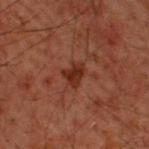Part of a total-body skin-imaging series; this lesion was reviewed on a skin check and was not flagged for biopsy. An algorithmic analysis of the crop reported an eccentricity of roughly 0.65 and a shape-asymmetry score of about 0.4 (0 = symmetric). And it measured a lesion color around L≈22 a*≈21 b*≈24 in CIELAB, roughly 8 lightness units darker than nearby skin, and a normalized border contrast of about 9. And it measured a border-irregularity index near 4/10, a color-variation rating of about 2/10, and a peripheral color-asymmetry measure near 0.5. On the upper back. About 2.5 mm across. Imaged with cross-polarized lighting. A region of skin cropped from a whole-body photographic capture, roughly 15 mm wide. A male subject, aged 58 to 62.